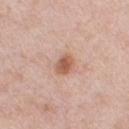The lesion was tiled from a total-body skin photograph and was not biopsied. A male patient, aged around 40. Approximately 3 mm at its widest. An algorithmic analysis of the crop reported a normalized lesion–skin contrast near 8. It also reported a border-irregularity index near 1/10, internal color variation of about 3 on a 0–10 scale, and radial color variation of about 0.5. It also reported a nevus-likeness score of about 90/100. Cropped from a total-body skin-imaging series; the visible field is about 15 mm. The lesion is on the chest. This is a white-light tile.